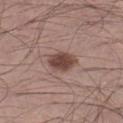Case summary:
- automated lesion analysis — about 13 CIELAB-L* units darker than the surrounding skin and a lesion-to-skin contrast of about 9.5 (normalized; higher = more distinct); a border-irregularity rating of about 2/10, a color-variation rating of about 3/10, and radial color variation of about 1; a nevus-likeness score of about 95/100 and lesion-presence confidence of about 100/100
- anatomic site — the left lower leg
- subject — male, aged 53–57
- acquisition — 15 mm crop, total-body photography